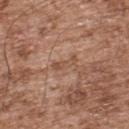workup — imaged on a skin check; not biopsied | imaging modality — 15 mm crop, total-body photography | subject — male, aged 53–57 | site — the upper back | illumination — white-light illumination.